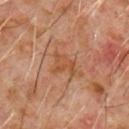Q: Was a biopsy performed?
A: imaged on a skin check; not biopsied
Q: Illumination type?
A: cross-polarized illumination
Q: What is the imaging modality?
A: ~15 mm tile from a whole-body skin photo
Q: What did automated image analysis measure?
A: a lesion area of about 5 mm², an eccentricity of roughly 0.75, and a shape-asymmetry score of about 0.45 (0 = symmetric); a border-irregularity index near 5.5/10, a within-lesion color-variation index near 2/10, and radial color variation of about 0.5; a classifier nevus-likeness of about 0/100
Q: What is the anatomic site?
A: the chest
Q: Who is the patient?
A: male, approximately 60 years of age
Q: What is the lesion's diameter?
A: ≈3 mm